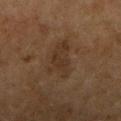<lesion>
<biopsy_status>not biopsied; imaged during a skin examination</biopsy_status>
<patient>
  <sex>female</sex>
  <age_approx>60</age_approx>
</patient>
<lighting>cross-polarized</lighting>
<image>
  <source>total-body photography crop</source>
  <field_of_view_mm>15</field_of_view_mm>
</image>
<site>arm</site>
</lesion>